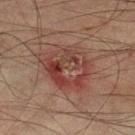workup=no biopsy performed (imaged during a skin exam) | illumination=cross-polarized | image source=15 mm crop, total-body photography | patient=male, about 75 years old | lesion diameter=≈6.5 mm | body site=the left lower leg | TBP lesion metrics=an average lesion color of about L≈35 a*≈20 b*≈21 (CIELAB), a lesion–skin lightness drop of about 7, and a normalized lesion–skin contrast near 7; internal color variation of about 8.5 on a 0–10 scale and peripheral color asymmetry of about 3; an automated nevus-likeness rating near 0 out of 100 and a lesion-detection confidence of about 100/100.